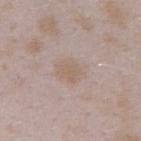lighting = white-light; site = the left forearm; image-analysis metrics = a border-irregularity index near 2/10, internal color variation of about 2.5 on a 0–10 scale, and a peripheral color-asymmetry measure near 1; imaging modality = ~15 mm crop, total-body skin-cancer survey; patient = female, in their mid-20s.